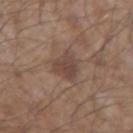image: ~15 mm crop, total-body skin-cancer survey
location: the arm
subject: male, in their mid-50s